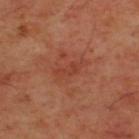- follow-up · catalogued during a skin exam; not biopsied
- patient · male, approximately 45 years of age
- image · total-body-photography crop, ~15 mm field of view
- tile lighting · cross-polarized
- anatomic site · the upper back